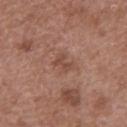Background:
This is a white-light tile. The subject is a male aged 48 to 52. A lesion tile, about 15 mm wide, cut from a 3D total-body photograph. On the mid back. Automated tile analysis of the lesion measured a mean CIELAB color near L≈47 a*≈21 b*≈27, a lesion–skin lightness drop of about 7, and a lesion-to-skin contrast of about 5.5 (normalized; higher = more distinct).